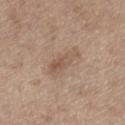imaging modality = total-body-photography crop, ~15 mm field of view | site = the left thigh | patient = male, aged around 70.